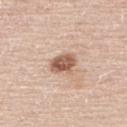Image and clinical context: An algorithmic analysis of the crop reported an eccentricity of roughly 0.65 and a symmetry-axis asymmetry near 0.2. And it measured a mean CIELAB color near L≈58 a*≈21 b*≈29 and about 15 CIELAB-L* units darker than the surrounding skin. The software also gave a border-irregularity rating of about 2/10, a color-variation rating of about 5/10, and a peripheral color-asymmetry measure near 1.5. Longest diameter approximately 3 mm. Imaged with white-light lighting. On the upper back. A male patient aged 58 to 62. A 15 mm close-up extracted from a 3D total-body photography capture.